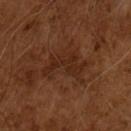The lesion was photographed on a routine skin check and not biopsied; there is no pathology result. About 5.5 mm across. The patient is a male roughly 65 years of age. A 15 mm close-up extracted from a 3D total-body photography capture.